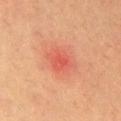Notes:
* follow-up: total-body-photography surveillance lesion; no biopsy
* patient: male, about 70 years old
* location: the chest
* image: 15 mm crop, total-body photography